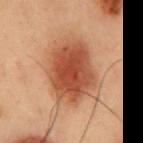{
  "biopsy_status": "not biopsied; imaged during a skin examination",
  "site": "chest",
  "lesion_size": {
    "long_diameter_mm_approx": 7.0
  },
  "lighting": "cross-polarized",
  "patient": {
    "sex": "male",
    "age_approx": 55
  },
  "image": {
    "source": "total-body photography crop",
    "field_of_view_mm": 15
  }
}